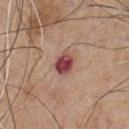The tile uses white-light illumination. From the chest. The patient is a male in their mid- to late 60s. A close-up tile cropped from a whole-body skin photograph, about 15 mm across.